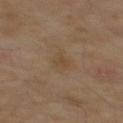Imaged during a routine full-body skin examination; the lesion was not biopsied and no histopathology is available. A male patient, aged 53–57. A roughly 15 mm field-of-view crop from a total-body skin photograph. The lesion-visualizer software estimated a border-irregularity index near 2/10, internal color variation of about 1.5 on a 0–10 scale, and radial color variation of about 0.5. And it measured a classifier nevus-likeness of about 0/100 and lesion-presence confidence of about 100/100. The lesion is located on the mid back. Imaged with cross-polarized lighting. Approximately 3 mm at its widest.